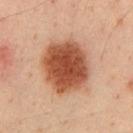workup: imaged on a skin check; not biopsied | anatomic site: the chest | subject: male, roughly 35 years of age | image-analysis metrics: an outline eccentricity of about 0.5 (0 = round, 1 = elongated) and two-axis asymmetry of about 0.1; a lesion–skin lightness drop of about 16; peripheral color asymmetry of about 1; an automated nevus-likeness rating near 100 out of 100 | lesion diameter: ≈6.5 mm | imaging modality: ~15 mm crop, total-body skin-cancer survey | illumination: cross-polarized.